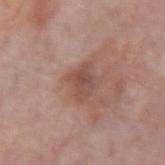Q: Was this lesion biopsied?
A: catalogued during a skin exam; not biopsied
Q: Who is the patient?
A: female, aged approximately 65
Q: Lesion location?
A: the right forearm
Q: What kind of image is this?
A: ~15 mm tile from a whole-body skin photo
Q: Lesion size?
A: ≈3.5 mm
Q: Illumination type?
A: white-light illumination
Q: What did automated image analysis measure?
A: a lesion area of about 6.5 mm² and a shape-asymmetry score of about 0.3 (0 = symmetric); a mean CIELAB color near L≈49 a*≈21 b*≈26, a lesion–skin lightness drop of about 9, and a normalized border contrast of about 6.5; a color-variation rating of about 3/10 and a peripheral color-asymmetry measure near 1